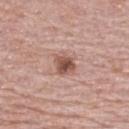Recorded during total-body skin imaging; not selected for excision or biopsy. The patient is a female aged 63–67. The total-body-photography lesion software estimated border irregularity of about 2 on a 0–10 scale and a color-variation rating of about 5/10. The analysis additionally found a detector confidence of about 100 out of 100 that the crop contains a lesion. About 2.5 mm across. A close-up tile cropped from a whole-body skin photograph, about 15 mm across. From the upper back.